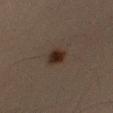Case summary:
• biopsy status — imaged on a skin check; not biopsied